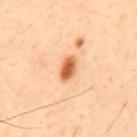Notes:
* anatomic site: the mid back
* automated metrics: a lesion color around L≈63 a*≈27 b*≈42 in CIELAB and a lesion-to-skin contrast of about 10 (normalized; higher = more distinct); a border-irregularity rating of about 1.5/10, a color-variation rating of about 5.5/10, and radial color variation of about 1.5; a classifier nevus-likeness of about 100/100 and a detector confidence of about 100 out of 100 that the crop contains a lesion
* lesion size: ≈3 mm
* patient: male, aged around 45
* image: ~15 mm tile from a whole-body skin photo
* tile lighting: cross-polarized illumination